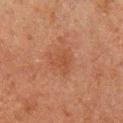Impression: No biopsy was performed on this lesion — it was imaged during a full skin examination and was not determined to be concerning.